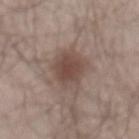The lesion was tiled from a total-body skin photograph and was not biopsied. Automated tile analysis of the lesion measured a lesion color around L≈47 a*≈16 b*≈22 in CIELAB, roughly 10 lightness units darker than nearby skin, and a normalized border contrast of about 7.5. The analysis additionally found a border-irregularity index near 2/10, a color-variation rating of about 3/10, and peripheral color asymmetry of about 1. Captured under white-light illumination. A 15 mm crop from a total-body photograph taken for skin-cancer surveillance. The subject is a male about 50 years old. Located on the lower back. The recorded lesion diameter is about 5 mm.